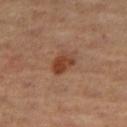Q: Was a biopsy performed?
A: catalogued during a skin exam; not biopsied
Q: How large is the lesion?
A: ~3 mm (longest diameter)
Q: Where on the body is the lesion?
A: the right thigh
Q: What is the imaging modality?
A: total-body-photography crop, ~15 mm field of view
Q: Illumination type?
A: cross-polarized
Q: Who is the patient?
A: female, aged 38–42